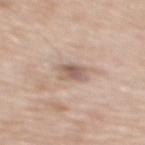patient — male, about 70 years old
location — the upper back
imaging modality — total-body-photography crop, ~15 mm field of view